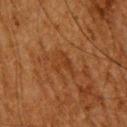Impression:
No biopsy was performed on this lesion — it was imaged during a full skin examination and was not determined to be concerning.
Image and clinical context:
A 15 mm close-up tile from a total-body photography series done for melanoma screening. The lesion is on the head or neck. The subject is a male aged 63 to 67.